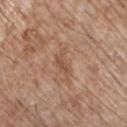<case>
  <biopsy_status>not biopsied; imaged during a skin examination</biopsy_status>
  <site>chest</site>
  <lesion_size>
    <long_diameter_mm_approx>3.5</long_diameter_mm_approx>
  </lesion_size>
  <automated_metrics>
    <area_mm2_approx>4.0</area_mm2_approx>
    <eccentricity>0.85</eccentricity>
    <shape_asymmetry>0.45</shape_asymmetry>
  </automated_metrics>
  <patient>
    <sex>male</sex>
    <age_approx>65</age_approx>
  </patient>
  <image>
    <source>total-body photography crop</source>
    <field_of_view_mm>15</field_of_view_mm>
  </image>
  <lighting>white-light</lighting>
</case>